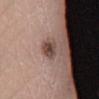Q: Was this lesion biopsied?
A: no biopsy performed (imaged during a skin exam)
Q: Automated lesion metrics?
A: a lesion area of about 5 mm² and a shape-asymmetry score of about 0.3 (0 = symmetric); a lesion–skin lightness drop of about 13; a border-irregularity index near 2.5/10, a within-lesion color-variation index near 5/10, and peripheral color asymmetry of about 1.5
Q: What are the patient's age and sex?
A: female, roughly 55 years of age
Q: Lesion location?
A: the left lower leg
Q: Illumination type?
A: white-light illumination
Q: How large is the lesion?
A: ≈2.5 mm
Q: What is the imaging modality?
A: ~15 mm crop, total-body skin-cancer survey